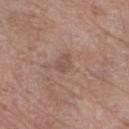Q: Is there a histopathology result?
A: imaged on a skin check; not biopsied
Q: Where on the body is the lesion?
A: the left lower leg
Q: What kind of image is this?
A: total-body-photography crop, ~15 mm field of view
Q: What lighting was used for the tile?
A: white-light
Q: What did automated image analysis measure?
A: a footprint of about 2.5 mm², an eccentricity of roughly 0.85, and a symmetry-axis asymmetry near 0.4; a lesion color around L≈50 a*≈19 b*≈24 in CIELAB, a lesion–skin lightness drop of about 7, and a lesion-to-skin contrast of about 5.5 (normalized; higher = more distinct); a border-irregularity index near 5/10 and internal color variation of about 0 on a 0–10 scale; an automated nevus-likeness rating near 0 out of 100 and lesion-presence confidence of about 100/100
Q: Who is the patient?
A: female, aged approximately 70
Q: How large is the lesion?
A: ~2.5 mm (longest diameter)